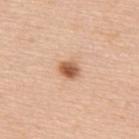{"lesion_size": {"long_diameter_mm_approx": 2.5}, "lighting": "white-light", "site": "upper back", "image": {"source": "total-body photography crop", "field_of_view_mm": 15}, "patient": {"sex": "male", "age_approx": 50}}